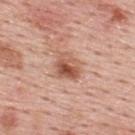Impression:
The lesion was photographed on a routine skin check and not biopsied; there is no pathology result.